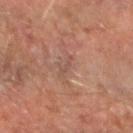Captured during whole-body skin photography for melanoma surveillance; the lesion was not biopsied. The recorded lesion diameter is about 2.5 mm. Cropped from a whole-body photographic skin survey; the tile spans about 15 mm. The subject is a male approximately 65 years of age. Imaged with cross-polarized lighting. On the right forearm.